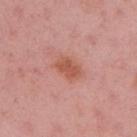Captured during whole-body skin photography for melanoma surveillance; the lesion was not biopsied. From the right upper arm. This image is a 15 mm lesion crop taken from a total-body photograph. The patient is a female about 35 years old. Measured at roughly 3.5 mm in maximum diameter. Automated tile analysis of the lesion measured a shape eccentricity near 0.8 and two-axis asymmetry of about 0.2. The software also gave an average lesion color of about L≈56 a*≈27 b*≈31 (CIELAB) and roughly 9 lightness units darker than nearby skin. The software also gave border irregularity of about 2 on a 0–10 scale, a within-lesion color-variation index near 2/10, and peripheral color asymmetry of about 0.5. It also reported a classifier nevus-likeness of about 85/100 and a detector confidence of about 100 out of 100 that the crop contains a lesion. Imaged with white-light lighting.